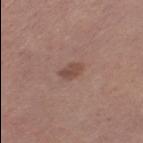Q: Who is the patient?
A: female, aged approximately 30
Q: What is the imaging modality?
A: 15 mm crop, total-body photography
Q: Where on the body is the lesion?
A: the left thigh